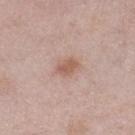Q: Was this lesion biopsied?
A: imaged on a skin check; not biopsied
Q: Patient demographics?
A: female, in their 60s
Q: What is the imaging modality?
A: ~15 mm crop, total-body skin-cancer survey
Q: Lesion location?
A: the left lower leg
Q: How was the tile lit?
A: white-light